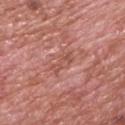lighting=white-light illumination | location=the upper back | diameter=about 3 mm | acquisition=~15 mm tile from a whole-body skin photo | patient=male, in their 70s | image-analysis metrics=a mean CIELAB color near L≈52 a*≈26 b*≈28 and about 7 CIELAB-L* units darker than the surrounding skin; a border-irregularity rating of about 5.5/10, a color-variation rating of about 0/10, and radial color variation of about 0; a classifier nevus-likeness of about 0/100 and a lesion-detection confidence of about 80/100.